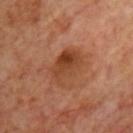{
  "biopsy_status": "not biopsied; imaged during a skin examination",
  "lesion_size": {
    "long_diameter_mm_approx": 4.5
  },
  "patient": {
    "sex": "male",
    "age_approx": 70
  },
  "automated_metrics": {
    "vs_skin_contrast_norm": 7.5,
    "border_irregularity_0_10": 3.0,
    "color_variation_0_10": 6.0,
    "peripheral_color_asymmetry": 2.5
  },
  "image": {
    "source": "total-body photography crop",
    "field_of_view_mm": 15
  },
  "site": "front of the torso",
  "lighting": "cross-polarized"
}